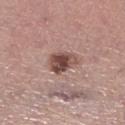Assessment:
Recorded during total-body skin imaging; not selected for excision or biopsy.
Background:
Located on the leg. The total-body-photography lesion software estimated a lesion color around L≈47 a*≈20 b*≈22 in CIELAB. The analysis additionally found border irregularity of about 2.5 on a 0–10 scale, a color-variation rating of about 6/10, and peripheral color asymmetry of about 2. The patient is a female about 60 years old. A lesion tile, about 15 mm wide, cut from a 3D total-body photograph. This is a white-light tile. About 3.5 mm across.